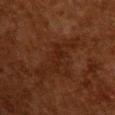Notes:
- follow-up: no biopsy performed (imaged during a skin exam)
- imaging modality: ~15 mm tile from a whole-body skin photo
- diameter: ≈4 mm
- body site: the upper back
- automated metrics: a lesion color around L≈18 a*≈18 b*≈23 in CIELAB, about 4 CIELAB-L* units darker than the surrounding skin, and a normalized border contrast of about 6; a border-irregularity index near 5.5/10, internal color variation of about 1 on a 0–10 scale, and peripheral color asymmetry of about 0; an automated nevus-likeness rating near 0 out of 100 and lesion-presence confidence of about 95/100
- subject: female, about 50 years old
- tile lighting: cross-polarized illumination